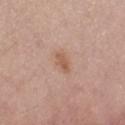Recorded during total-body skin imaging; not selected for excision or biopsy. Captured under white-light illumination. On the leg. A male subject approximately 65 years of age. Measured at roughly 2.5 mm in maximum diameter. A 15 mm close-up extracted from a 3D total-body photography capture.